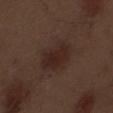No biopsy was performed on this lesion — it was imaged during a full skin examination and was not determined to be concerning. The lesion is on the front of the torso. Cropped from a whole-body photographic skin survey; the tile spans about 15 mm. The total-body-photography lesion software estimated a shape eccentricity near 0.7 and a symmetry-axis asymmetry near 0.15. The analysis additionally found an automated nevus-likeness rating near 75 out of 100 and a detector confidence of about 100 out of 100 that the crop contains a lesion. Imaged with white-light lighting. Approximately 5 mm at its widest. The subject is a male about 70 years old.